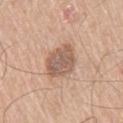Q: Was a biopsy performed?
A: no biopsy performed (imaged during a skin exam)
Q: Lesion location?
A: the right thigh
Q: What is the lesion's diameter?
A: ~4 mm (longest diameter)
Q: What is the imaging modality?
A: 15 mm crop, total-body photography
Q: Who is the patient?
A: male, aged around 70